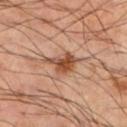  biopsy_status: not biopsied; imaged during a skin examination
  patient:
    sex: male
    age_approx: 50
  image:
    source: total-body photography crop
    field_of_view_mm: 15
  lesion_size:
    long_diameter_mm_approx: 4.5
  site: left lower leg
  lighting: cross-polarized
  automated_metrics:
    cielab_L: 50
    cielab_a: 23
    cielab_b: 33
    vs_skin_contrast_norm: 9.0
    border_irregularity_0_10: 5.0
    color_variation_0_10: 4.5
    peripheral_color_asymmetry: 1.5
    nevus_likeness_0_100: 80
    lesion_detection_confidence_0_100: 100